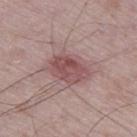Part of a total-body skin-imaging series; this lesion was reviewed on a skin check and was not flagged for biopsy. About 5 mm across. The patient is a male about 75 years old. A roughly 15 mm field-of-view crop from a total-body skin photograph. This is a white-light tile. Located on the left thigh.